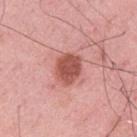A male subject, aged 33–37.
The lesion is located on the arm.
A 15 mm crop from a total-body photograph taken for skin-cancer surveillance.